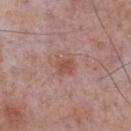notes — catalogued during a skin exam; not biopsied | lesion diameter — ≈3 mm | body site — the chest | TBP lesion metrics — an area of roughly 6 mm² and two-axis asymmetry of about 0.2; a lesion color around L≈52 a*≈22 b*≈26 in CIELAB, a lesion–skin lightness drop of about 7, and a lesion-to-skin contrast of about 6.5 (normalized; higher = more distinct); a classifier nevus-likeness of about 5/100 and lesion-presence confidence of about 100/100 | image — ~15 mm crop, total-body skin-cancer survey | patient — male, aged 73–77 | lighting — white-light illumination.